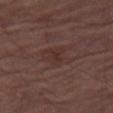<lesion>
  <biopsy_status>not biopsied; imaged during a skin examination</biopsy_status>
  <image>
    <source>total-body photography crop</source>
    <field_of_view_mm>15</field_of_view_mm>
  </image>
  <lighting>white-light</lighting>
  <lesion_size>
    <long_diameter_mm_approx>2.5</long_diameter_mm_approx>
  </lesion_size>
  <automated_metrics>
    <area_mm2_approx>4.0</area_mm2_approx>
    <eccentricity>0.55</eccentricity>
    <shape_asymmetry>0.4</shape_asymmetry>
    <lesion_detection_confidence_0_100>100</lesion_detection_confidence_0_100>
  </automated_metrics>
  <site>right thigh</site>
  <patient>
    <sex>male</sex>
    <age_approx>75</age_approx>
  </patient>
</lesion>